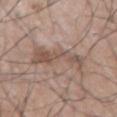The lesion was photographed on a routine skin check and not biopsied; there is no pathology result.
Captured under white-light illumination.
Automated image analysis of the tile measured a border-irregularity index near 7.5/10, a within-lesion color-variation index near 4.5/10, and a peripheral color-asymmetry measure near 1.5. The software also gave a classifier nevus-likeness of about 0/100 and a detector confidence of about 100 out of 100 that the crop contains a lesion.
Located on the arm.
A 15 mm crop from a total-body photograph taken for skin-cancer surveillance.
Longest diameter approximately 6.5 mm.
A male patient, approximately 75 years of age.